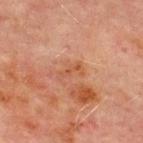About 3 mm across.
On the front of the torso.
A region of skin cropped from a whole-body photographic capture, roughly 15 mm wide.
A male subject aged around 70.
An algorithmic analysis of the crop reported an area of roughly 3 mm², an eccentricity of roughly 0.9, and a symmetry-axis asymmetry near 0.5.
Imaged with cross-polarized lighting.